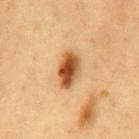biopsy status — total-body-photography surveillance lesion; no biopsy | anatomic site — the chest | size — ≈4.5 mm | image — 15 mm crop, total-body photography | tile lighting — cross-polarized illumination | patient — male, aged around 60.